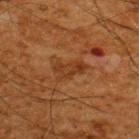Q: How was this image acquired?
A: ~15 mm crop, total-body skin-cancer survey
Q: What are the patient's age and sex?
A: male, in their mid-60s
Q: Lesion location?
A: the upper back